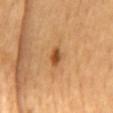- notes · no biopsy performed (imaged during a skin exam)
- subject · male, aged around 65
- TBP lesion metrics · two-axis asymmetry of about 0.4; lesion-presence confidence of about 100/100
- imaging modality · ~15 mm tile from a whole-body skin photo
- body site · the back
- lighting · cross-polarized illumination
- lesion size · ~2.5 mm (longest diameter)